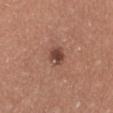| feature | finding |
|---|---|
| workup | total-body-photography surveillance lesion; no biopsy |
| location | the leg |
| acquisition | ~15 mm crop, total-body skin-cancer survey |
| lighting | white-light |
| automated lesion analysis | an area of roughly 4 mm² and an outline eccentricity of about 0.65 (0 = round, 1 = elongated) |
| patient | female, aged 38 to 42 |
| size | ≈2.5 mm |